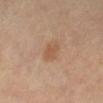workup — catalogued during a skin exam; not biopsied | imaging modality — 15 mm crop, total-body photography | subject — female, in their mid- to late 60s | lesion diameter — ≈3 mm | tile lighting — cross-polarized illumination | location — the right lower leg | TBP lesion metrics — a footprint of about 4.5 mm² and a shape-asymmetry score of about 0.25 (0 = symmetric); a normalized border contrast of about 6; a within-lesion color-variation index near 1.5/10 and peripheral color asymmetry of about 0.5; an automated nevus-likeness rating near 30 out of 100 and a detector confidence of about 100 out of 100 that the crop contains a lesion.